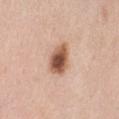follow-up: imaged on a skin check; not biopsied
image source: total-body-photography crop, ~15 mm field of view
body site: the leg
illumination: white-light
subject: female, aged 48–52